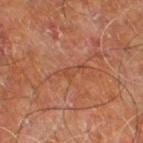<lesion>
<biopsy_status>not biopsied; imaged during a skin examination</biopsy_status>
<site>right leg</site>
<lesion_size>
  <long_diameter_mm_approx>3.5</long_diameter_mm_approx>
</lesion_size>
<lighting>cross-polarized</lighting>
<image>
  <source>total-body photography crop</source>
  <field_of_view_mm>15</field_of_view_mm>
</image>
<patient>
  <sex>male</sex>
  <age_approx>60</age_approx>
</patient>
</lesion>